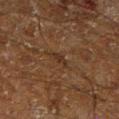<record>
<lighting>cross-polarized</lighting>
<site>right lower leg</site>
<image>
  <source>total-body photography crop</source>
  <field_of_view_mm>15</field_of_view_mm>
</image>
<automated_metrics>
  <area_mm2_approx>2.5</area_mm2_approx>
  <eccentricity>0.9</eccentricity>
  <shape_asymmetry>0.35</shape_asymmetry>
  <cielab_L>23</cielab_L>
  <cielab_a>14</cielab_a>
  <cielab_b>22</cielab_b>
  <vs_skin_darker_L>5.0</vs_skin_darker_L>
  <vs_skin_contrast_norm>6.0</vs_skin_contrast_norm>
  <border_irregularity_0_10>3.5</border_irregularity_0_10>
  <color_variation_0_10>0.0</color_variation_0_10>
  <peripheral_color_asymmetry>0.0</peripheral_color_asymmetry>
  <nevus_likeness_0_100>0</nevus_likeness_0_100>
  <lesion_detection_confidence_0_100>60</lesion_detection_confidence_0_100>
</automated_metrics>
<lesion_size>
  <long_diameter_mm_approx>2.5</long_diameter_mm_approx>
</lesion_size>
<patient>
  <sex>male</sex>
  <age_approx>60</age_approx>
</patient>
</record>